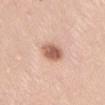Clinical impression:
Recorded during total-body skin imaging; not selected for excision or biopsy.
Acquisition and patient details:
This is a white-light tile. A female patient aged 28 to 32. The lesion is located on the abdomen. A 15 mm close-up tile from a total-body photography series done for melanoma screening. About 3.5 mm across.